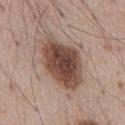| key | value |
|---|---|
| follow-up | imaged on a skin check; not biopsied |
| image-analysis metrics | a shape eccentricity near 0.8 and two-axis asymmetry of about 0.2; a lesion color around L≈46 a*≈18 b*≈25 in CIELAB, about 16 CIELAB-L* units darker than the surrounding skin, and a normalized lesion–skin contrast near 11.5; a border-irregularity rating of about 2/10, a within-lesion color-variation index near 5.5/10, and peripheral color asymmetry of about 1.5; a nevus-likeness score of about 80/100 |
| subject | male, aged approximately 55 |
| site | the abdomen |
| size | about 7 mm |
| lighting | white-light illumination |
| acquisition | total-body-photography crop, ~15 mm field of view |